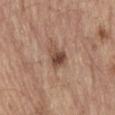Clinical impression: Imaged during a routine full-body skin examination; the lesion was not biopsied and no histopathology is available. Image and clinical context: A 15 mm close-up tile from a total-body photography series done for melanoma screening. The lesion is on the abdomen. The subject is a male aged 68 to 72.